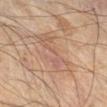Background:
Approximately 5.5 mm at its widest. This is a cross-polarized tile. A roughly 15 mm field-of-view crop from a total-body skin photograph. The lesion is located on the right lower leg. The subject is a male aged approximately 65.